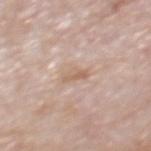{"biopsy_status": "not biopsied; imaged during a skin examination", "lesion_size": {"long_diameter_mm_approx": 2.5}, "site": "mid back", "image": {"source": "total-body photography crop", "field_of_view_mm": 15}, "lighting": "white-light", "patient": {"sex": "male", "age_approx": 75}}